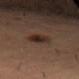notes: catalogued during a skin exam; not biopsied | anatomic site: the right thigh | subject: male, in their mid-50s | image: 15 mm crop, total-body photography.